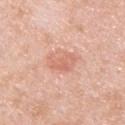image:
  source: total-body photography crop
  field_of_view_mm: 15
automated_metrics:
  peripheral_color_asymmetry: 1.0
site: upper back
lesion_size:
  long_diameter_mm_approx: 4.0
patient:
  sex: male
  age_approx: 45